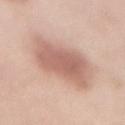Q: Was a biopsy performed?
A: catalogued during a skin exam; not biopsied
Q: Automated lesion metrics?
A: a mean CIELAB color near L≈62 a*≈21 b*≈27, roughly 12 lightness units darker than nearby skin, and a lesion-to-skin contrast of about 7 (normalized; higher = more distinct)
Q: What are the patient's age and sex?
A: female, about 50 years old
Q: What is the anatomic site?
A: the abdomen
Q: How large is the lesion?
A: about 7 mm
Q: What kind of image is this?
A: 15 mm crop, total-body photography
Q: What lighting was used for the tile?
A: white-light